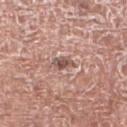Impression:
This lesion was catalogued during total-body skin photography and was not selected for biopsy.
Acquisition and patient details:
The tile uses white-light illumination. The lesion is on the right lower leg. Automated image analysis of the tile measured an average lesion color of about L≈52 a*≈19 b*≈23 (CIELAB), about 12 CIELAB-L* units darker than the surrounding skin, and a normalized border contrast of about 8. And it measured a border-irregularity index near 3/10 and a peripheral color-asymmetry measure near 0.5. A 15 mm close-up tile from a total-body photography series done for melanoma screening. A male subject, aged around 75.